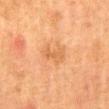No biopsy was performed on this lesion — it was imaged during a full skin examination and was not determined to be concerning.
An algorithmic analysis of the crop reported a lesion color around L≈52 a*≈22 b*≈37 in CIELAB and about 6 CIELAB-L* units darker than the surrounding skin. It also reported border irregularity of about 3.5 on a 0–10 scale, internal color variation of about 1.5 on a 0–10 scale, and peripheral color asymmetry of about 0.5. The software also gave a lesion-detection confidence of about 100/100.
A close-up tile cropped from a whole-body skin photograph, about 15 mm across.
A female patient aged approximately 50.
The lesion is on the abdomen.
Captured under cross-polarized illumination.